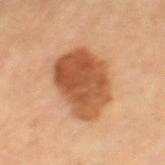No biopsy was performed on this lesion — it was imaged during a full skin examination and was not determined to be concerning. The total-body-photography lesion software estimated a lesion color around L≈52 a*≈25 b*≈36 in CIELAB and a lesion-to-skin contrast of about 10 (normalized; higher = more distinct). A close-up tile cropped from a whole-body skin photograph, about 15 mm across. On the leg. The lesion's longest dimension is about 6.5 mm. A female patient, aged 68–72. This is a cross-polarized tile.